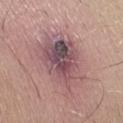No biopsy was performed on this lesion — it was imaged during a full skin examination and was not determined to be concerning.
Captured under white-light illumination.
The lesion is located on the right forearm.
A male subject aged 63 to 67.
A lesion tile, about 15 mm wide, cut from a 3D total-body photograph.